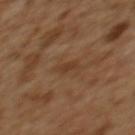Part of a total-body skin-imaging series; this lesion was reviewed on a skin check and was not flagged for biopsy.
The subject is a female aged approximately 55.
The lesion is located on the upper back.
A roughly 15 mm field-of-view crop from a total-body skin photograph.